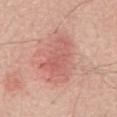Clinical impression: No biopsy was performed on this lesion — it was imaged during a full skin examination and was not determined to be concerning. Image and clinical context: A 15 mm close-up tile from a total-body photography series done for melanoma screening. From the mid back. A male patient approximately 70 years of age. The tile uses white-light illumination. Approximately 5 mm at its widest.